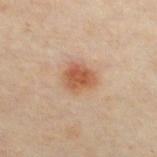Q: Was this lesion biopsied?
A: no biopsy performed (imaged during a skin exam)
Q: Who is the patient?
A: male, aged around 50
Q: What kind of image is this?
A: total-body-photography crop, ~15 mm field of view
Q: What is the anatomic site?
A: the chest